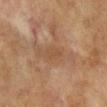Assessment:
No biopsy was performed on this lesion — it was imaged during a full skin examination and was not determined to be concerning.
Acquisition and patient details:
The lesion is located on the chest. This is a cross-polarized tile. About 2.5 mm across. A female patient, aged 58 to 62. The total-body-photography lesion software estimated a mean CIELAB color near L≈45 a*≈18 b*≈31, about 5 CIELAB-L* units darker than the surrounding skin, and a normalized lesion–skin contrast near 4.5. The analysis additionally found border irregularity of about 3 on a 0–10 scale, a color-variation rating of about 0.5/10, and a peripheral color-asymmetry measure near 0. Cropped from a total-body skin-imaging series; the visible field is about 15 mm.